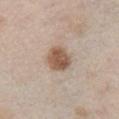Findings:
- follow-up · catalogued during a skin exam; not biopsied
- image source · 15 mm crop, total-body photography
- automated metrics · an outline eccentricity of about 0.5 (0 = round, 1 = elongated) and a shape-asymmetry score of about 0.15 (0 = symmetric); a mean CIELAB color near L≈55 a*≈17 b*≈28 and a lesion–skin lightness drop of about 14
- patient · male, about 55 years old
- location · the right upper arm
- lesion diameter · ≈3.5 mm